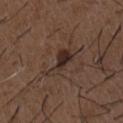The lesion was photographed on a routine skin check and not biopsied; there is no pathology result.
A close-up tile cropped from a whole-body skin photograph, about 15 mm across.
A male patient, about 50 years old.
Captured under white-light illumination.
From the chest.
Approximately 4.5 mm at its widest.